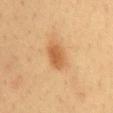notes: total-body-photography surveillance lesion; no biopsy
image: total-body-photography crop, ~15 mm field of view
patient: male, in their mid- to late 30s
tile lighting: cross-polarized illumination
diameter: about 3.5 mm
automated lesion analysis: an area of roughly 7 mm², a shape eccentricity near 0.8, and a shape-asymmetry score of about 0.2 (0 = symmetric); a detector confidence of about 100 out of 100 that the crop contains a lesion
body site: the mid back